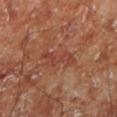biopsy_status: not biopsied; imaged during a skin examination
patient:
  sex: male
  age_approx: 70
lighting: cross-polarized
automated_metrics:
  area_mm2_approx: 5.5
  eccentricity: 0.95
  shape_asymmetry: 0.65
  vs_skin_darker_L: 6.0
  vs_skin_contrast_norm: 5.0
  border_irregularity_0_10: 8.5
  peripheral_color_asymmetry: 0.0
image:
  source: total-body photography crop
  field_of_view_mm: 15
site: left lower leg